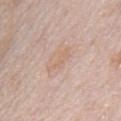No biopsy was performed on this lesion — it was imaged during a full skin examination and was not determined to be concerning.
Imaged with white-light lighting.
The lesion is located on the chest.
Automated tile analysis of the lesion measured a footprint of about 3.5 mm², an outline eccentricity of about 0.95 (0 = round, 1 = elongated), and a shape-asymmetry score of about 0.55 (0 = symmetric). It also reported a nevus-likeness score of about 0/100 and a detector confidence of about 100 out of 100 that the crop contains a lesion.
A roughly 15 mm field-of-view crop from a total-body skin photograph.
A male patient aged 38–42.
The lesion's longest dimension is about 3.5 mm.